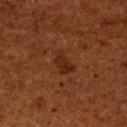- follow-up — imaged on a skin check; not biopsied
- lesion size — ~3 mm (longest diameter)
- illumination — cross-polarized
- subject — male, about 60 years old
- body site — the right upper arm
- image source — total-body-photography crop, ~15 mm field of view